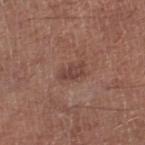biopsy_status: not biopsied; imaged during a skin examination
site: right lower leg
image:
  source: total-body photography crop
  field_of_view_mm: 15
lesion_size:
  long_diameter_mm_approx: 3.0
patient:
  sex: male
  age_approx: 70
lighting: white-light